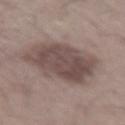Recorded during total-body skin imaging; not selected for excision or biopsy. Located on the right thigh. The recorded lesion diameter is about 7.5 mm. Cropped from a whole-body photographic skin survey; the tile spans about 15 mm. The tile uses white-light illumination. The patient is a male aged around 20.